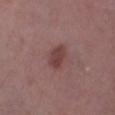Part of a total-body skin-imaging series; this lesion was reviewed on a skin check and was not flagged for biopsy. A male subject, aged around 55. Automated image analysis of the tile measured a lesion area of about 5.5 mm² and a shape-asymmetry score of about 0.2 (0 = symmetric). The software also gave a border-irregularity index near 2/10, a within-lesion color-variation index near 2.5/10, and peripheral color asymmetry of about 1. And it measured an automated nevus-likeness rating near 40 out of 100. Located on the right lower leg. Approximately 3.5 mm at its widest. A roughly 15 mm field-of-view crop from a total-body skin photograph.